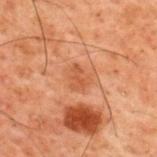Part of a total-body skin-imaging series; this lesion was reviewed on a skin check and was not flagged for biopsy.